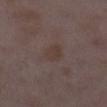workup = imaged on a skin check; not biopsied
site = the right lower leg
subject = female, approximately 30 years of age
lighting = white-light
acquisition = 15 mm crop, total-body photography
lesion size = about 2.5 mm